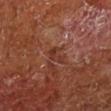Imaged during a routine full-body skin examination; the lesion was not biopsied and no histopathology is available. The lesion is located on the right lower leg. A male patient, aged approximately 65. Cropped from a total-body skin-imaging series; the visible field is about 15 mm. The recorded lesion diameter is about 3 mm.